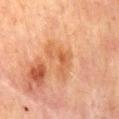Imaged during a routine full-body skin examination; the lesion was not biopsied and no histopathology is available.